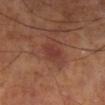Part of a total-body skin-imaging series; this lesion was reviewed on a skin check and was not flagged for biopsy.
An algorithmic analysis of the crop reported an outline eccentricity of about 0.8 (0 = round, 1 = elongated) and two-axis asymmetry of about 0.35. The software also gave border irregularity of about 3 on a 0–10 scale, a within-lesion color-variation index near 0.5/10, and radial color variation of about 0. The software also gave a classifier nevus-likeness of about 35/100 and lesion-presence confidence of about 100/100.
From the right lower leg.
A lesion tile, about 15 mm wide, cut from a 3D total-body photograph.
Imaged with cross-polarized lighting.
Measured at roughly 2.5 mm in maximum diameter.
A male patient, approximately 70 years of age.